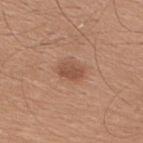Assessment: Imaged during a routine full-body skin examination; the lesion was not biopsied and no histopathology is available. Acquisition and patient details: The tile uses white-light illumination. A 15 mm crop from a total-body photograph taken for skin-cancer surveillance. The recorded lesion diameter is about 3 mm. From the upper back. Automated image analysis of the tile measured an area of roughly 5 mm², an outline eccentricity of about 0.75 (0 = round, 1 = elongated), and a symmetry-axis asymmetry near 0.2. The software also gave a border-irregularity rating of about 2/10, a within-lesion color-variation index near 2.5/10, and radial color variation of about 1. A male patient aged 28 to 32.